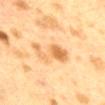| key | value |
|---|---|
| follow-up | total-body-photography surveillance lesion; no biopsy |
| automated lesion analysis | a classifier nevus-likeness of about 35/100 and lesion-presence confidence of about 100/100 |
| illumination | cross-polarized |
| site | the mid back |
| imaging modality | ~15 mm crop, total-body skin-cancer survey |
| subject | female, aged 38 to 42 |
| lesion diameter | ~5 mm (longest diameter) |